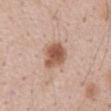Assessment:
No biopsy was performed on this lesion — it was imaged during a full skin examination and was not determined to be concerning.
Context:
The lesion-visualizer software estimated an area of roughly 8.5 mm² and an outline eccentricity of about 0.5 (0 = round, 1 = elongated). It also reported a lesion color around L≈55 a*≈21 b*≈29 in CIELAB and a lesion-to-skin contrast of about 9.5 (normalized; higher = more distinct). The software also gave a color-variation rating of about 4/10 and peripheral color asymmetry of about 1.5. The tile uses white-light illumination. A male subject aged 58 to 62. A region of skin cropped from a whole-body photographic capture, roughly 15 mm wide. The recorded lesion diameter is about 3.5 mm. On the abdomen.